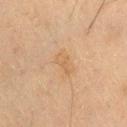Recorded during total-body skin imaging; not selected for excision or biopsy. The lesion-visualizer software estimated a lesion color around L≈50 a*≈15 b*≈32 in CIELAB, about 5 CIELAB-L* units darker than the surrounding skin, and a lesion-to-skin contrast of about 4.5 (normalized; higher = more distinct). The software also gave an automated nevus-likeness rating near 0 out of 100 and a detector confidence of about 100 out of 100 that the crop contains a lesion. A 15 mm close-up extracted from a 3D total-body photography capture. The subject is a male in their 70s. The tile uses cross-polarized illumination. Measured at roughly 3 mm in maximum diameter. From the right thigh.